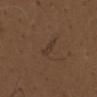<tbp_lesion>
<biopsy_status>not biopsied; imaged during a skin examination</biopsy_status>
<image>
  <source>total-body photography crop</source>
  <field_of_view_mm>15</field_of_view_mm>
</image>
<lesion_size>
  <long_diameter_mm_approx>3.0</long_diameter_mm_approx>
</lesion_size>
<site>chest</site>
<automated_metrics>
  <area_mm2_approx>2.5</area_mm2_approx>
  <shape_asymmetry>0.4</shape_asymmetry>
  <vs_skin_darker_L>5.0</vs_skin_darker_L>
  <vs_skin_contrast_norm>5.5</vs_skin_contrast_norm>
  <border_irregularity_0_10>4.5</border_irregularity_0_10>
  <color_variation_0_10>0.0</color_variation_0_10>
  <peripheral_color_asymmetry>0.0</peripheral_color_asymmetry>
  <nevus_likeness_0_100>0</nevus_likeness_0_100>
  <lesion_detection_confidence_0_100>55</lesion_detection_confidence_0_100>
</automated_metrics>
<patient>
  <sex>male</sex>
  <age_approx>70</age_approx>
</patient>
</tbp_lesion>